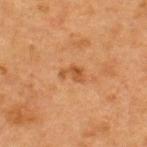Q: Was a biopsy performed?
A: no biopsy performed (imaged during a skin exam)
Q: Who is the patient?
A: female, approximately 40 years of age
Q: How was this image acquired?
A: ~15 mm crop, total-body skin-cancer survey
Q: How was the tile lit?
A: cross-polarized illumination
Q: Lesion size?
A: ~3 mm (longest diameter)
Q: Where on the body is the lesion?
A: the upper back